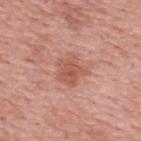Imaged during a routine full-body skin examination; the lesion was not biopsied and no histopathology is available. Longest diameter approximately 3.5 mm. On the upper back. This image is a 15 mm lesion crop taken from a total-body photograph. A female patient, aged 38 to 42. The tile uses white-light illumination.